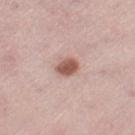Impression:
Captured during whole-body skin photography for melanoma surveillance; the lesion was not biopsied.
Background:
A lesion tile, about 15 mm wide, cut from a 3D total-body photograph. The lesion's longest dimension is about 2.5 mm. A female subject, about 45 years old. On the left thigh.